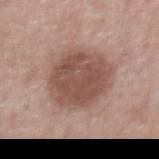Assessment:
The lesion was tiled from a total-body skin photograph and was not biopsied.
Context:
Imaged with white-light lighting. Approximately 6.5 mm at its widest. A male patient aged 63–67. The lesion is located on the mid back. A region of skin cropped from a whole-body photographic capture, roughly 15 mm wide.